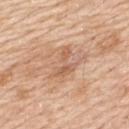Clinical summary: Approximately 4 mm at its widest. The total-body-photography lesion software estimated an average lesion color of about L≈60 a*≈21 b*≈33 (CIELAB), roughly 8 lightness units darker than nearby skin, and a normalized border contrast of about 5.5. Imaged with white-light lighting. From the upper back. A male patient aged 58–62. This image is a 15 mm lesion crop taken from a total-body photograph.